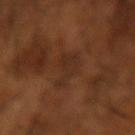No biopsy was performed on this lesion — it was imaged during a full skin examination and was not determined to be concerning. Captured under cross-polarized illumination. A 15 mm crop from a total-body photograph taken for skin-cancer surveillance. About 4 mm across. The lesion is on the right forearm. A male patient aged 63–67.